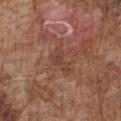follow-up: catalogued during a skin exam; not biopsied
illumination: white-light illumination
acquisition: total-body-photography crop, ~15 mm field of view
subject: male, approximately 75 years of age
site: the chest
lesion diameter: about 3.5 mm
image-analysis metrics: an area of roughly 5 mm² and a shape-asymmetry score of about 0.5 (0 = symmetric); a mean CIELAB color near L≈42 a*≈21 b*≈27 and a normalized border contrast of about 5; an automated nevus-likeness rating near 0 out of 100